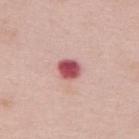This lesion was catalogued during total-body skin photography and was not selected for biopsy. A female patient aged 63 to 67. The tile uses white-light illumination. This image is a 15 mm lesion crop taken from a total-body photograph. Located on the back. Longest diameter approximately 2.5 mm.